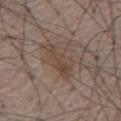workup: no biopsy performed (imaged during a skin exam) | anatomic site: the front of the torso | imaging modality: ~15 mm tile from a whole-body skin photo | subject: male, in their 80s | lighting: white-light | diameter: ≈5.5 mm.